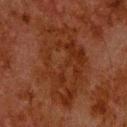biopsy status = imaged on a skin check; not biopsied | acquisition = total-body-photography crop, ~15 mm field of view | location = the head or neck | subject = male, about 80 years old.